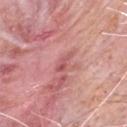This lesion was catalogued during total-body skin photography and was not selected for biopsy. Imaged with white-light lighting. A close-up tile cropped from a whole-body skin photograph, about 15 mm across. Located on the chest. A male patient approximately 80 years of age. Automated tile analysis of the lesion measured a lesion color around L≈55 a*≈31 b*≈23 in CIELAB.